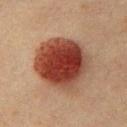Case summary:
– workup · no biopsy performed (imaged during a skin exam)
– anatomic site · the front of the torso
– patient · female, aged approximately 40
– acquisition · ~15 mm tile from a whole-body skin photo